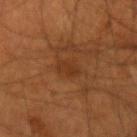Findings:
– workup: catalogued during a skin exam; not biopsied
– lighting: cross-polarized illumination
– image: ~15 mm tile from a whole-body skin photo
– location: the right forearm
– patient: male, approximately 55 years of age
– automated metrics: a footprint of about 4 mm² and a shape eccentricity near 0.7; a mean CIELAB color near L≈32 a*≈21 b*≈32, a lesion–skin lightness drop of about 5, and a normalized border contrast of about 5